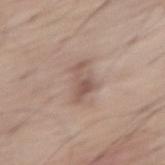notes = total-body-photography surveillance lesion; no biopsy
image = ~15 mm crop, total-body skin-cancer survey
anatomic site = the right thigh
size = ~4 mm (longest diameter)
lighting = white-light illumination
patient = male, approximately 70 years of age
TBP lesion metrics = an area of roughly 6 mm², an eccentricity of roughly 0.9, and a symmetry-axis asymmetry near 0.5; a mean CIELAB color near L≈54 a*≈16 b*≈24, about 10 CIELAB-L* units darker than the surrounding skin, and a normalized border contrast of about 6.5; internal color variation of about 2.5 on a 0–10 scale and peripheral color asymmetry of about 1